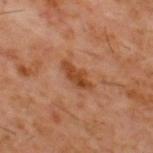biopsy_status: not biopsied; imaged during a skin examination
patient:
  sex: male
  age_approx: 60
image:
  source: total-body photography crop
  field_of_view_mm: 15
lighting: cross-polarized
lesion_size:
  long_diameter_mm_approx: 4.0
site: upper back